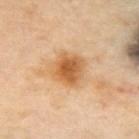Part of a total-body skin-imaging series; this lesion was reviewed on a skin check and was not flagged for biopsy.
Approximately 3.5 mm at its widest.
A female patient, in their mid-40s.
Located on the upper back.
Captured under cross-polarized illumination.
A lesion tile, about 15 mm wide, cut from a 3D total-body photograph.
Automated image analysis of the tile measured a footprint of about 11 mm², a shape eccentricity near 0.2, and two-axis asymmetry of about 0.15. And it measured a lesion color around L≈61 a*≈22 b*≈42 in CIELAB, roughly 12 lightness units darker than nearby skin, and a lesion-to-skin contrast of about 8.5 (normalized; higher = more distinct). It also reported border irregularity of about 2 on a 0–10 scale and peripheral color asymmetry of about 1.5.